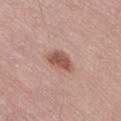| key | value |
|---|---|
| notes | catalogued during a skin exam; not biopsied |
| illumination | white-light |
| imaging modality | ~15 mm crop, total-body skin-cancer survey |
| site | the lower back |
| patient | male, aged 68 to 72 |
| image-analysis metrics | a shape eccentricity near 0.8 and a symmetry-axis asymmetry near 0.25; an average lesion color of about L≈53 a*≈22 b*≈27 (CIELAB), roughly 12 lightness units darker than nearby skin, and a normalized border contrast of about 8.5; an automated nevus-likeness rating near 90 out of 100 and a lesion-detection confidence of about 100/100 |
| lesion size | about 3.5 mm |